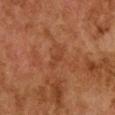The patient is a female approximately 60 years of age. The tile uses cross-polarized illumination. On the chest. A close-up tile cropped from a whole-body skin photograph, about 15 mm across. Measured at roughly 2.5 mm in maximum diameter.